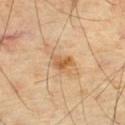notes — catalogued during a skin exam; not biopsied | patient — male, aged approximately 70 | imaging modality — total-body-photography crop, ~15 mm field of view | illumination — cross-polarized illumination | automated metrics — an eccentricity of roughly 0.65; lesion-presence confidence of about 100/100 | location — the leg | diameter — ~3 mm (longest diameter).